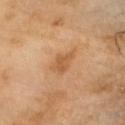The lesion was photographed on a routine skin check and not biopsied; there is no pathology result.
An algorithmic analysis of the crop reported an area of roughly 5 mm², an outline eccentricity of about 0.65 (0 = round, 1 = elongated), and a shape-asymmetry score of about 0.3 (0 = symmetric). The software also gave an average lesion color of about L≈52 a*≈20 b*≈37 (CIELAB), roughly 8 lightness units darker than nearby skin, and a normalized lesion–skin contrast near 6. The analysis additionally found a border-irregularity rating of about 3/10, internal color variation of about 1.5 on a 0–10 scale, and radial color variation of about 0.5. It also reported lesion-presence confidence of about 100/100.
On the head or neck.
Cropped from a whole-body photographic skin survey; the tile spans about 15 mm.
Imaged with cross-polarized lighting.
The patient is a male in their 60s.
The lesion's longest dimension is about 3 mm.